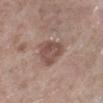Q: Was a biopsy performed?
A: catalogued during a skin exam; not biopsied
Q: Where on the body is the lesion?
A: the right lower leg
Q: What are the patient's age and sex?
A: male, aged approximately 75
Q: How was this image acquired?
A: 15 mm crop, total-body photography